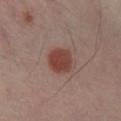biopsy_status: not biopsied; imaged during a skin examination
site: right lower leg
lesion_size:
  long_diameter_mm_approx: 3.5
lighting: cross-polarized
image:
  source: total-body photography crop
  field_of_view_mm: 15
automated_metrics:
  area_mm2_approx: 8.0
  eccentricity: 0.25
  border_irregularity_0_10: 1.5
  color_variation_0_10: 2.5
  peripheral_color_asymmetry: 0.5
  nevus_likeness_0_100: 100
patient:
  sex: male
  age_approx: 40